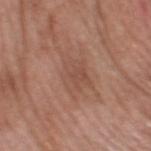Impression: The lesion was tiled from a total-body skin photograph and was not biopsied. Context: The lesion-visualizer software estimated an area of roughly 11 mm² and two-axis asymmetry of about 0.35. It also reported a lesion color around L≈50 a*≈21 b*≈27 in CIELAB and a normalized border contrast of about 4.5. Located on the head or neck. A male patient, about 60 years old. A 15 mm close-up extracted from a 3D total-body photography capture. Approximately 5.5 mm at its widest. The tile uses white-light illumination.